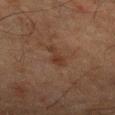{
  "biopsy_status": "not biopsied; imaged during a skin examination",
  "automated_metrics": {
    "area_mm2_approx": 4.0,
    "shape_asymmetry": 0.5,
    "cielab_L": 28,
    "cielab_a": 16,
    "cielab_b": 23,
    "vs_skin_darker_L": 5.0,
    "vs_skin_contrast_norm": 6.0,
    "border_irregularity_0_10": 5.5,
    "color_variation_0_10": 1.0,
    "peripheral_color_asymmetry": 0.0,
    "nevus_likeness_0_100": 0,
    "lesion_detection_confidence_0_100": 100
  },
  "image": {
    "source": "total-body photography crop",
    "field_of_view_mm": 15
  },
  "lesion_size": {
    "long_diameter_mm_approx": 2.5
  },
  "patient": {
    "sex": "male",
    "age_approx": 65
  },
  "site": "left forearm"
}